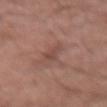Context:
The lesion is on the right upper arm. A male patient, aged around 70. A lesion tile, about 15 mm wide, cut from a 3D total-body photograph. This is a white-light tile. Longest diameter approximately 3.5 mm. The lesion-visualizer software estimated an area of roughly 3.5 mm², an eccentricity of roughly 0.9, and a shape-asymmetry score of about 0.5 (0 = symmetric). The software also gave a mean CIELAB color near L≈47 a*≈21 b*≈24. The software also gave a border-irregularity index near 5.5/10, internal color variation of about 1 on a 0–10 scale, and peripheral color asymmetry of about 0.5.